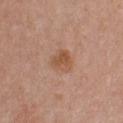Impression: Recorded during total-body skin imaging; not selected for excision or biopsy. Image and clinical context: Approximately 3 mm at its widest. Automated image analysis of the tile measured an area of roughly 6 mm², an eccentricity of roughly 0.35, and two-axis asymmetry of about 0.3. And it measured a mean CIELAB color near L≈53 a*≈22 b*≈32, a lesion–skin lightness drop of about 8, and a normalized border contrast of about 7. And it measured a lesion-detection confidence of about 100/100. A female subject, roughly 50 years of age. A 15 mm close-up tile from a total-body photography series done for melanoma screening. The lesion is located on the chest.